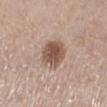No biopsy was performed on this lesion — it was imaged during a full skin examination and was not determined to be concerning.
On the left lower leg.
Measured at roughly 4 mm in maximum diameter.
Captured under white-light illumination.
A close-up tile cropped from a whole-body skin photograph, about 15 mm across.
The subject is a female roughly 65 years of age.